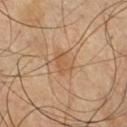Impression:
This lesion was catalogued during total-body skin photography and was not selected for biopsy.
Clinical summary:
A region of skin cropped from a whole-body photographic capture, roughly 15 mm wide. A male subject, aged 38 to 42. The recorded lesion diameter is about 3 mm. From the chest. An algorithmic analysis of the crop reported a lesion area of about 4.5 mm², an outline eccentricity of about 0.75 (0 = round, 1 = elongated), and a symmetry-axis asymmetry near 0.3. The software also gave a lesion–skin lightness drop of about 7 and a lesion-to-skin contrast of about 5.5 (normalized; higher = more distinct). It also reported a border-irregularity index near 3/10, internal color variation of about 2 on a 0–10 scale, and radial color variation of about 0.5. The software also gave a lesion-detection confidence of about 100/100.